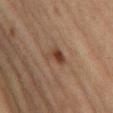The lesion was photographed on a routine skin check and not biopsied; there is no pathology result. Located on the left thigh. A close-up tile cropped from a whole-body skin photograph, about 15 mm across. The subject is a female aged around 50.